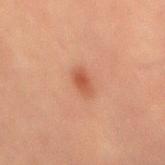Assessment: Captured during whole-body skin photography for melanoma surveillance; the lesion was not biopsied. Background: The patient is a male aged 43–47. Cropped from a total-body skin-imaging series; the visible field is about 15 mm. On the back. Approximately 3 mm at its widest. The tile uses cross-polarized illumination.